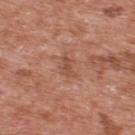biopsy status: imaged on a skin check; not biopsied | size: about 2.5 mm | acquisition: total-body-photography crop, ~15 mm field of view | patient: male, in their 70s | automated metrics: a footprint of about 2.5 mm², an outline eccentricity of about 0.9 (0 = round, 1 = elongated), and two-axis asymmetry of about 0.45 | site: the upper back.